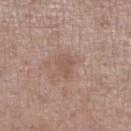Captured during whole-body skin photography for melanoma surveillance; the lesion was not biopsied.
This image is a 15 mm lesion crop taken from a total-body photograph.
The tile uses white-light illumination.
About 3 mm across.
The lesion is located on the right lower leg.
A male subject in their mid- to late 50s.
Automated tile analysis of the lesion measured a border-irregularity rating of about 3.5/10, internal color variation of about 2 on a 0–10 scale, and peripheral color asymmetry of about 0.5.